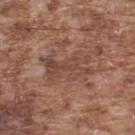Notes:
– workup — no biopsy performed (imaged during a skin exam)
– subject — male, aged 73 to 77
– lighting — white-light illumination
– site — the upper back
– image — total-body-photography crop, ~15 mm field of view